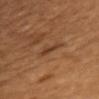From the chest. A lesion tile, about 15 mm wide, cut from a 3D total-body photograph. The patient is a male aged 53–57. Imaged with cross-polarized lighting.